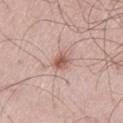Case summary:
• workup: catalogued during a skin exam; not biopsied
• tile lighting: white-light
• lesion size: ~2.5 mm (longest diameter)
• automated metrics: an automated nevus-likeness rating near 85 out of 100 and lesion-presence confidence of about 100/100
• acquisition: 15 mm crop, total-body photography
• patient: male, aged approximately 55
• location: the leg